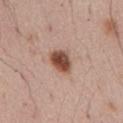This lesion was catalogued during total-body skin photography and was not selected for biopsy.